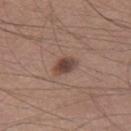Captured during whole-body skin photography for melanoma surveillance; the lesion was not biopsied. This image is a 15 mm lesion crop taken from a total-body photograph. Measured at roughly 3 mm in maximum diameter. From the leg. This is a white-light tile. The patient is a male approximately 45 years of age.